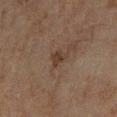Findings:
- notes: imaged on a skin check; not biopsied
- acquisition: total-body-photography crop, ~15 mm field of view
- subject: male, roughly 45 years of age
- body site: the left forearm